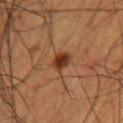Q: Is there a histopathology result?
A: catalogued during a skin exam; not biopsied
Q: What is the anatomic site?
A: the left upper arm
Q: What kind of image is this?
A: ~15 mm tile from a whole-body skin photo
Q: Patient demographics?
A: male, in their 50s